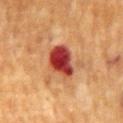No biopsy was performed on this lesion — it was imaged during a full skin examination and was not determined to be concerning. A male patient, about 70 years old. A close-up tile cropped from a whole-body skin photograph, about 15 mm across. Located on the mid back.